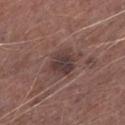Impression: Captured during whole-body skin photography for melanoma surveillance; the lesion was not biopsied. Image and clinical context: On the left lower leg. This is a white-light tile. A region of skin cropped from a whole-body photographic capture, roughly 15 mm wide. A male subject approximately 75 years of age. An algorithmic analysis of the crop reported an outline eccentricity of about 0.7 (0 = round, 1 = elongated) and a symmetry-axis asymmetry near 0.2. The software also gave a border-irregularity index near 2.5/10 and a within-lesion color-variation index near 4/10.